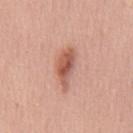Part of a total-body skin-imaging series; this lesion was reviewed on a skin check and was not flagged for biopsy. A male patient aged 53–57. Approximately 5.5 mm at its widest. The lesion is located on the chest. The lesion-visualizer software estimated a border-irregularity rating of about 4.5/10, a within-lesion color-variation index near 6/10, and radial color variation of about 2. It also reported a nevus-likeness score of about 95/100 and lesion-presence confidence of about 100/100. This is a white-light tile. Cropped from a total-body skin-imaging series; the visible field is about 15 mm.